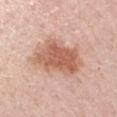<lesion>
  <biopsy_status>not biopsied; imaged during a skin examination</biopsy_status>
  <image>
    <source>total-body photography crop</source>
    <field_of_view_mm>15</field_of_view_mm>
  </image>
  <site>arm</site>
  <lighting>white-light</lighting>
  <patient>
    <sex>male</sex>
    <age_approx>60</age_approx>
  </patient>
</lesion>